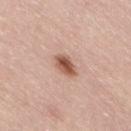biopsy_status: not biopsied; imaged during a skin examination
image:
  source: total-body photography crop
  field_of_view_mm: 15
lighting: white-light
site: leg
patient:
  sex: female
  age_approx: 65
automated_metrics:
  border_irregularity_0_10: 1.5
  color_variation_0_10: 3.5
  peripheral_color_asymmetry: 1.0
  lesion_detection_confidence_0_100: 100
lesion_size:
  long_diameter_mm_approx: 3.0